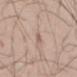Recorded during total-body skin imaging; not selected for excision or biopsy.
A 15 mm close-up extracted from a 3D total-body photography capture.
From the left upper arm.
A male subject roughly 40 years of age.